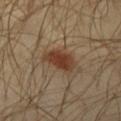{
  "lesion_size": {
    "long_diameter_mm_approx": 4.0
  },
  "site": "upper back",
  "automated_metrics": {
    "area_mm2_approx": 8.5,
    "eccentricity": 0.75,
    "shape_asymmetry": 0.25,
    "cielab_L": 37,
    "cielab_a": 18,
    "cielab_b": 28
  },
  "lighting": "cross-polarized",
  "image": {
    "source": "total-body photography crop",
    "field_of_view_mm": 15
  },
  "patient": {
    "sex": "male",
    "age_approx": 30
  }
}